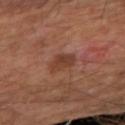Part of a total-body skin-imaging series; this lesion was reviewed on a skin check and was not flagged for biopsy. Captured under cross-polarized illumination. A region of skin cropped from a whole-body photographic capture, roughly 15 mm wide. An algorithmic analysis of the crop reported a lesion color around L≈41 a*≈23 b*≈29 in CIELAB and a normalized lesion–skin contrast near 7. And it measured a border-irregularity index near 2/10, internal color variation of about 3 on a 0–10 scale, and radial color variation of about 1. The lesion is on the right forearm. The recorded lesion diameter is about 3 mm.